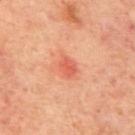Background:
A male subject, about 70 years old. Approximately 2.5 mm at its widest. A roughly 15 mm field-of-view crop from a total-body skin photograph. The lesion is on the mid back.